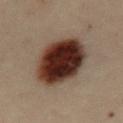A 15 mm close-up extracted from a 3D total-body photography capture. On the abdomen. A female patient, in their 30s.